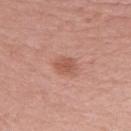On the right forearm. A 15 mm close-up extracted from a 3D total-body photography capture. Automated image analysis of the tile measured a mean CIELAB color near L≈55 a*≈25 b*≈29, a lesion–skin lightness drop of about 9, and a normalized lesion–skin contrast near 6.5. The analysis additionally found a within-lesion color-variation index near 2/10 and a peripheral color-asymmetry measure near 0.5. Longest diameter approximately 3 mm. Imaged with white-light lighting. A female subject, aged around 40.